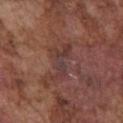Q: Is there a histopathology result?
A: total-body-photography surveillance lesion; no biopsy
Q: What did automated image analysis measure?
A: an area of roughly 7 mm² and an outline eccentricity of about 0.85 (0 = round, 1 = elongated); a color-variation rating of about 4/10 and radial color variation of about 0.5; a classifier nevus-likeness of about 0/100 and a lesion-detection confidence of about 95/100
Q: How was this image acquired?
A: 15 mm crop, total-body photography
Q: Patient demographics?
A: male, in their mid- to late 70s
Q: Illumination type?
A: white-light illumination
Q: Where on the body is the lesion?
A: the chest
Q: Lesion size?
A: ≈4.5 mm